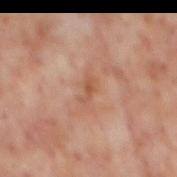Findings:
* follow-up — catalogued during a skin exam; not biopsied
* location — the mid back
* illumination — cross-polarized illumination
* patient — male, approximately 65 years of age
* acquisition — ~15 mm crop, total-body skin-cancer survey